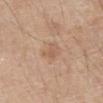{"biopsy_status": "not biopsied; imaged during a skin examination", "lighting": "white-light", "site": "abdomen", "image": {"source": "total-body photography crop", "field_of_view_mm": 15}, "automated_metrics": {"area_mm2_approx": 4.0, "eccentricity": 0.85, "cielab_L": 58, "cielab_a": 19, "cielab_b": 32, "vs_skin_darker_L": 7.0}, "lesion_size": {"long_diameter_mm_approx": 3.0}, "patient": {"sex": "male", "age_approx": 80}}